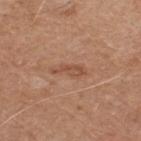lesion size — ~4 mm (longest diameter); anatomic site — the back; image source — 15 mm crop, total-body photography; patient — male, aged 63–67.